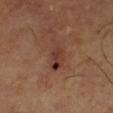Imaged during a routine full-body skin examination; the lesion was not biopsied and no histopathology is available.
A lesion tile, about 15 mm wide, cut from a 3D total-body photograph.
The lesion-visualizer software estimated a lesion color around L≈36 a*≈22 b*≈25 in CIELAB, roughly 8 lightness units darker than nearby skin, and a lesion-to-skin contrast of about 7.5 (normalized; higher = more distinct). It also reported a border-irregularity index near 3.5/10, a color-variation rating of about 6.5/10, and radial color variation of about 2. The analysis additionally found a classifier nevus-likeness of about 0/100 and lesion-presence confidence of about 100/100.
Imaged with cross-polarized lighting.
The patient is a male aged around 65.
Measured at roughly 4.5 mm in maximum diameter.
From the right lower leg.